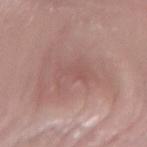The patient is a female aged 38–42. The lesion is on the arm. The tile uses white-light illumination. Automated tile analysis of the lesion measured a footprint of about 9 mm², an outline eccentricity of about 0.9 (0 = round, 1 = elongated), and a shape-asymmetry score of about 0.4 (0 = symmetric). A roughly 15 mm field-of-view crop from a total-body skin photograph.